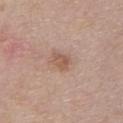{
  "lesion_size": {
    "long_diameter_mm_approx": 3.0
  },
  "patient": {
    "sex": "male",
    "age_approx": 40
  },
  "image": {
    "source": "total-body photography crop",
    "field_of_view_mm": 15
  },
  "site": "chest",
  "automated_metrics": {
    "nevus_likeness_0_100": 30,
    "lesion_detection_confidence_0_100": 100
  },
  "lighting": "white-light"
}